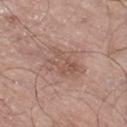notes: catalogued during a skin exam; not biopsied | body site: the leg | subject: male, aged around 70 | lesion size: ≈4.5 mm | imaging modality: total-body-photography crop, ~15 mm field of view | tile lighting: white-light.